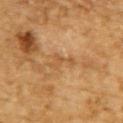The lesion was tiled from a total-body skin photograph and was not biopsied.
Captured under cross-polarized illumination.
On the upper back.
The recorded lesion diameter is about 3.5 mm.
A male patient, aged approximately 85 or older.
A lesion tile, about 15 mm wide, cut from a 3D total-body photograph.